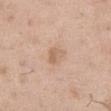| feature | finding |
|---|---|
| patient | male, in their 50s |
| site | the right thigh |
| image | ~15 mm crop, total-body skin-cancer survey |
| lesion diameter | ~3 mm (longest diameter) |
| illumination | white-light |
| automated lesion analysis | a mean CIELAB color near L≈63 a*≈18 b*≈31 and roughly 8 lightness units darker than nearby skin |